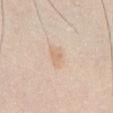Q: Was this lesion biopsied?
A: catalogued during a skin exam; not biopsied
Q: Where on the body is the lesion?
A: the abdomen
Q: Illumination type?
A: white-light
Q: What kind of image is this?
A: total-body-photography crop, ~15 mm field of view
Q: What are the patient's age and sex?
A: male, in their mid-30s
Q: What is the lesion's diameter?
A: about 3 mm